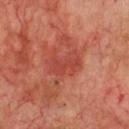biopsy status = catalogued during a skin exam; not biopsied
anatomic site = the chest
image source = ~15 mm crop, total-body skin-cancer survey
patient = male, about 70 years old